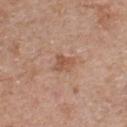Clinical impression: Imaged during a routine full-body skin examination; the lesion was not biopsied and no histopathology is available. Acquisition and patient details: Automated image analysis of the tile measured a footprint of about 4 mm², an eccentricity of roughly 0.6, and a shape-asymmetry score of about 0.35 (0 = symmetric). And it measured a border-irregularity index near 3.5/10 and internal color variation of about 2 on a 0–10 scale. The software also gave a classifier nevus-likeness of about 0/100 and a detector confidence of about 100 out of 100 that the crop contains a lesion. A 15 mm crop from a total-body photograph taken for skin-cancer surveillance. This is a white-light tile. About 2.5 mm across. A male patient approximately 70 years of age. On the front of the torso.